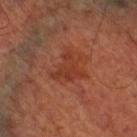The lesion was tiled from a total-body skin photograph and was not biopsied.
The lesion's longest dimension is about 4 mm.
A 15 mm crop from a total-body photograph taken for skin-cancer surveillance.
The patient is in their mid-60s.
The lesion is on the right lower leg.